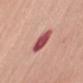Background:
On the right upper arm. A region of skin cropped from a whole-body photographic capture, roughly 15 mm wide. A female patient about 65 years old. This is a white-light tile. About 3.5 mm across.